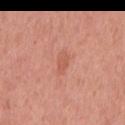biopsy status — catalogued during a skin exam; not biopsied | location — the mid back | subject — male, in their mid-50s | acquisition — ~15 mm crop, total-body skin-cancer survey.